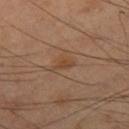Clinical impression: Captured during whole-body skin photography for melanoma surveillance; the lesion was not biopsied. Acquisition and patient details: The tile uses cross-polarized illumination. The patient is a male about 70 years old. The lesion is located on the right thigh. This image is a 15 mm lesion crop taken from a total-body photograph.